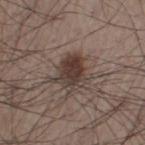This lesion was catalogued during total-body skin photography and was not selected for biopsy. Located on the left thigh. Approximately 4.5 mm at its widest. A roughly 15 mm field-of-view crop from a total-body skin photograph. The patient is a male in their mid-60s.